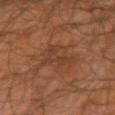This lesion was catalogued during total-body skin photography and was not selected for biopsy.
This image is a 15 mm lesion crop taken from a total-body photograph.
A male subject, aged approximately 65.
The lesion is on the arm.